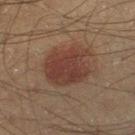follow-up — no biopsy performed (imaged during a skin exam) | image — 15 mm crop, total-body photography | diameter — ≈5.5 mm | site — the left thigh | patient — male, aged 38–42.